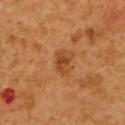No biopsy was performed on this lesion — it was imaged during a full skin examination and was not determined to be concerning. The subject is a female aged 53 to 57. From the back. A 15 mm close-up tile from a total-body photography series done for melanoma screening. Longest diameter approximately 3 mm. Captured under cross-polarized illumination. The total-body-photography lesion software estimated a shape eccentricity near 0.75 and a shape-asymmetry score of about 0.25 (0 = symmetric). It also reported about 9 CIELAB-L* units darker than the surrounding skin and a normalized lesion–skin contrast near 7. It also reported a color-variation rating of about 4/10 and radial color variation of about 1.5.